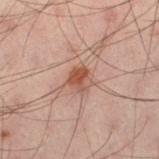notes — no biopsy performed (imaged during a skin exam) | imaging modality — total-body-photography crop, ~15 mm field of view | location — the left thigh | subject — male, aged 38 to 42 | automated metrics — a mean CIELAB color near L≈41 a*≈19 b*≈24 and a lesion-to-skin contrast of about 8 (normalized; higher = more distinct); a border-irregularity rating of about 2.5/10 and a within-lesion color-variation index near 4.5/10.